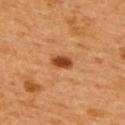Assessment:
Recorded during total-body skin imaging; not selected for excision or biopsy.
Image and clinical context:
The recorded lesion diameter is about 2.5 mm. A male patient, aged approximately 50. A roughly 15 mm field-of-view crop from a total-body skin photograph. Imaged with cross-polarized lighting. The lesion is on the upper back.